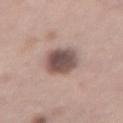Assessment: Captured during whole-body skin photography for melanoma surveillance; the lesion was not biopsied. Image and clinical context: Cropped from a whole-body photographic skin survey; the tile spans about 15 mm. The subject is a female aged 38–42. Measured at roughly 5 mm in maximum diameter. Captured under white-light illumination. The lesion is on the back. Automated tile analysis of the lesion measured an area of roughly 13 mm², an outline eccentricity of about 0.8 (0 = round, 1 = elongated), and two-axis asymmetry of about 0.15. The analysis additionally found roughly 16 lightness units darker than nearby skin. The software also gave a border-irregularity rating of about 1.5/10, a within-lesion color-variation index near 5/10, and a peripheral color-asymmetry measure near 1.5.